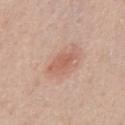Findings:
– follow-up · no biopsy performed (imaged during a skin exam)
– illumination · white-light
– imaging modality · ~15 mm tile from a whole-body skin photo
– subject · male, aged around 45
– automated metrics · a lesion area of about 7 mm², an eccentricity of roughly 0.85, and two-axis asymmetry of about 0.25; border irregularity of about 3 on a 0–10 scale and a within-lesion color-variation index near 2/10
– location · the front of the torso
– lesion diameter · ~4 mm (longest diameter)